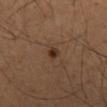<tbp_lesion>
<biopsy_status>not biopsied; imaged during a skin examination</biopsy_status>
<patient>
  <sex>male</sex>
  <age_approx>50</age_approx>
</patient>
<site>mid back</site>
<image>
  <source>total-body photography crop</source>
  <field_of_view_mm>15</field_of_view_mm>
</image>
</tbp_lesion>